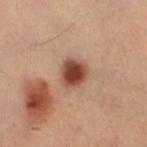The lesion was photographed on a routine skin check and not biopsied; there is no pathology result. The lesion is located on the leg. A roughly 15 mm field-of-view crop from a total-body skin photograph. This is a cross-polarized tile. A female patient aged 33–37. The recorded lesion diameter is about 3.5 mm. An algorithmic analysis of the crop reported a color-variation rating of about 5.5/10. The analysis additionally found a classifier nevus-likeness of about 100/100 and a lesion-detection confidence of about 100/100.